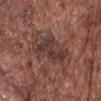The lesion was tiled from a total-body skin photograph and was not biopsied.
Longest diameter approximately 5 mm.
Cropped from a whole-body photographic skin survey; the tile spans about 15 mm.
The tile uses white-light illumination.
A male patient, aged approximately 75.
On the chest.
Automated tile analysis of the lesion measured a footprint of about 15 mm² and an outline eccentricity of about 0.65 (0 = round, 1 = elongated). It also reported a lesion–skin lightness drop of about 8 and a lesion-to-skin contrast of about 8 (normalized; higher = more distinct). It also reported a color-variation rating of about 5.5/10 and peripheral color asymmetry of about 1.5. The analysis additionally found lesion-presence confidence of about 95/100.